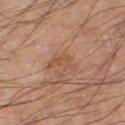Q: Was this lesion biopsied?
A: total-body-photography surveillance lesion; no biopsy
Q: How was the tile lit?
A: cross-polarized illumination
Q: How large is the lesion?
A: ≈3.5 mm
Q: Where on the body is the lesion?
A: the left thigh
Q: What is the imaging modality?
A: ~15 mm crop, total-body skin-cancer survey
Q: What are the patient's age and sex?
A: male, aged 63–67